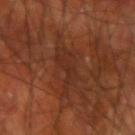Impression:
Captured during whole-body skin photography for melanoma surveillance; the lesion was not biopsied.
Context:
A male patient, aged 58–62. Imaged with cross-polarized lighting. The lesion is located on the right upper arm. Automated tile analysis of the lesion measured an area of roughly 8.5 mm² and a shape eccentricity near 0.8. And it measured border irregularity of about 6.5 on a 0–10 scale, internal color variation of about 2 on a 0–10 scale, and radial color variation of about 0.5. And it measured a nevus-likeness score of about 0/100. A close-up tile cropped from a whole-body skin photograph, about 15 mm across. The recorded lesion diameter is about 4.5 mm.